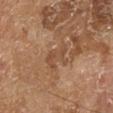Captured during whole-body skin photography for melanoma surveillance; the lesion was not biopsied. Longest diameter approximately 3.5 mm. A 15 mm close-up extracted from a 3D total-body photography capture. The total-body-photography lesion software estimated a border-irregularity index near 6/10, a within-lesion color-variation index near 0.5/10, and radial color variation of about 0. The analysis additionally found a classifier nevus-likeness of about 0/100 and a detector confidence of about 100 out of 100 that the crop contains a lesion. The subject is a male in their mid-60s. The lesion is on the right lower leg. Captured under cross-polarized illumination.